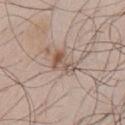Notes:
– follow-up · no biopsy performed (imaged during a skin exam)